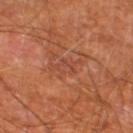This lesion was catalogued during total-body skin photography and was not selected for biopsy.
This image is a 15 mm lesion crop taken from a total-body photograph.
The lesion's longest dimension is about 3.5 mm.
The lesion is located on the leg.
The patient is a male aged approximately 65.